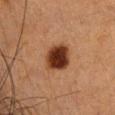Findings:
- biopsy status: total-body-photography surveillance lesion; no biopsy
- body site: the head or neck
- subject: female, in their 40s
- image: total-body-photography crop, ~15 mm field of view